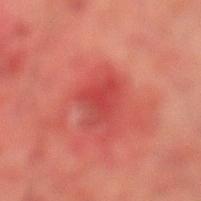notes: total-body-photography surveillance lesion; no biopsy | patient: male, in their mid-70s | image source: ~15 mm tile from a whole-body skin photo | illumination: cross-polarized illumination | site: the leg.